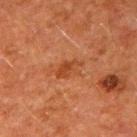Acquisition and patient details:
A lesion tile, about 15 mm wide, cut from a 3D total-body photograph. Automated image analysis of the tile measured a lesion–skin lightness drop of about 7 and a lesion-to-skin contrast of about 6.5 (normalized; higher = more distinct). And it measured a classifier nevus-likeness of about 5/100 and a detector confidence of about 100 out of 100 that the crop contains a lesion. The patient is a male about 60 years old. The lesion is located on the right upper arm. Approximately 3.5 mm at its widest. This is a cross-polarized tile.